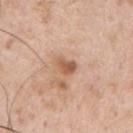<lesion>
<biopsy_status>not biopsied; imaged during a skin examination</biopsy_status>
<patient>
  <sex>male</sex>
  <age_approx>55</age_approx>
</patient>
<site>left upper arm</site>
<image>
  <source>total-body photography crop</source>
  <field_of_view_mm>15</field_of_view_mm>
</image>
</lesion>